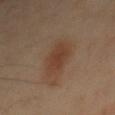Imaged during a routine full-body skin examination; the lesion was not biopsied and no histopathology is available.
The tile uses cross-polarized illumination.
The lesion is located on the mid back.
The lesion-visualizer software estimated a border-irregularity index near 3/10 and a within-lesion color-variation index near 2.5/10. The software also gave a nevus-likeness score of about 100/100.
The subject is a male aged around 55.
A 15 mm close-up tile from a total-body photography series done for melanoma screening.